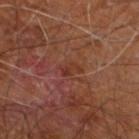Clinical impression: The lesion was photographed on a routine skin check and not biopsied; there is no pathology result. Clinical summary: On the right leg. Longest diameter approximately 2.5 mm. The tile uses cross-polarized illumination. Automated image analysis of the tile measured a footprint of about 3 mm², an outline eccentricity of about 0.8 (0 = round, 1 = elongated), and two-axis asymmetry of about 0.4. The analysis additionally found an average lesion color of about L≈34 a*≈24 b*≈27 (CIELAB), roughly 5 lightness units darker than nearby skin, and a normalized lesion–skin contrast near 5. It also reported a border-irregularity rating of about 5/10. The software also gave an automated nevus-likeness rating near 0 out of 100 and a lesion-detection confidence of about 100/100. A 15 mm close-up tile from a total-body photography series done for melanoma screening. A male patient, approximately 60 years of age.